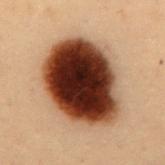Clinical impression: Imaged during a routine full-body skin examination; the lesion was not biopsied and no histopathology is available.